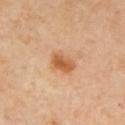No biopsy was performed on this lesion — it was imaged during a full skin examination and was not determined to be concerning. The lesion is on the arm. The subject is a male aged around 55. The total-body-photography lesion software estimated a mean CIELAB color near L≈59 a*≈23 b*≈40, a lesion–skin lightness drop of about 11, and a normalized lesion–skin contrast near 8. And it measured border irregularity of about 1.5 on a 0–10 scale, a color-variation rating of about 4.5/10, and radial color variation of about 1.5. The analysis additionally found a detector confidence of about 100 out of 100 that the crop contains a lesion. This is a cross-polarized tile. About 3 mm across. Cropped from a whole-body photographic skin survey; the tile spans about 15 mm.